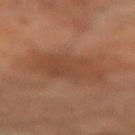Impression:
No biopsy was performed on this lesion — it was imaged during a full skin examination and was not determined to be concerning.
Context:
Imaged with cross-polarized lighting. The subject is a male approximately 70 years of age. Approximately 6 mm at its widest. Cropped from a total-body skin-imaging series; the visible field is about 15 mm. The lesion is located on the left forearm.